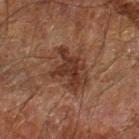Assessment:
The lesion was photographed on a routine skin check and not biopsied; there is no pathology result.
Background:
Imaged with cross-polarized lighting. This image is a 15 mm lesion crop taken from a total-body photograph. A male subject aged approximately 65. The lesion is on the arm.